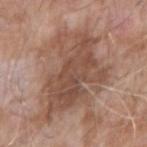Notes:
– biopsy status · imaged on a skin check; not biopsied
– image source · total-body-photography crop, ~15 mm field of view
– diameter · ≈10 mm
– patient · male, aged around 75
– body site · the right forearm
– automated lesion analysis · a footprint of about 39 mm² and an eccentricity of roughly 0.75
– illumination · white-light illumination